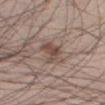Automated image analysis of the tile measured an area of roughly 6.5 mm², an outline eccentricity of about 0.65 (0 = round, 1 = elongated), and two-axis asymmetry of about 0.4.
The lesion's longest dimension is about 3.5 mm.
From the left thigh.
The patient is a male aged 53 to 57.
A close-up tile cropped from a whole-body skin photograph, about 15 mm across.